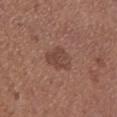This lesion was catalogued during total-body skin photography and was not selected for biopsy. A male subject roughly 75 years of age. Captured under white-light illumination. Approximately 3.5 mm at its widest. A roughly 15 mm field-of-view crop from a total-body skin photograph. An algorithmic analysis of the crop reported a footprint of about 7.5 mm², a shape eccentricity near 0.6, and two-axis asymmetry of about 0.2. And it measured an average lesion color of about L≈44 a*≈20 b*≈24 (CIELAB), roughly 7 lightness units darker than nearby skin, and a normalized border contrast of about 6. It also reported a border-irregularity index near 2/10, internal color variation of about 2.5 on a 0–10 scale, and a peripheral color-asymmetry measure near 1. The lesion is located on the left lower leg.